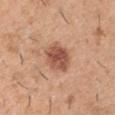notes — catalogued during a skin exam; not biopsied
image — total-body-photography crop, ~15 mm field of view
site — the right upper arm
subject — male, aged around 40